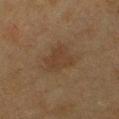The lesion was tiled from a total-body skin photograph and was not biopsied. The subject is a female aged approximately 40. Measured at roughly 4.5 mm in maximum diameter. Automated image analysis of the tile measured an area of roughly 10 mm², a shape eccentricity near 0.7, and two-axis asymmetry of about 0.3. The lesion is located on the chest. Imaged with cross-polarized lighting. A region of skin cropped from a whole-body photographic capture, roughly 15 mm wide.